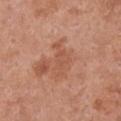A female patient aged 63 to 67.
Imaged with white-light lighting.
Cropped from a whole-body photographic skin survey; the tile spans about 15 mm.
Automated tile analysis of the lesion measured an area of roughly 6 mm² and an eccentricity of roughly 0.9. The software also gave a mean CIELAB color near L≈54 a*≈25 b*≈32, a lesion–skin lightness drop of about 7, and a normalized lesion–skin contrast near 5. The software also gave a border-irregularity index near 6.5/10, a color-variation rating of about 1.5/10, and radial color variation of about 0.5.
The recorded lesion diameter is about 4.5 mm.
From the chest.